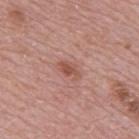biopsy status = catalogued during a skin exam; not biopsied | lesion size = ≈2.5 mm | location = the mid back | lighting = white-light illumination | acquisition = total-body-photography crop, ~15 mm field of view | patient = male, about 70 years old.